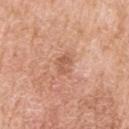{"biopsy_status": "not biopsied; imaged during a skin examination", "site": "left upper arm", "patient": {"sex": "male", "age_approx": 60}, "image": {"source": "total-body photography crop", "field_of_view_mm": 15}, "lighting": "white-light"}